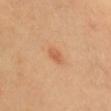The lesion was tiled from a total-body skin photograph and was not biopsied. A female patient, aged approximately 40. Captured under cross-polarized illumination. About 2.5 mm across. The lesion is located on the head or neck. A close-up tile cropped from a whole-body skin photograph, about 15 mm across.